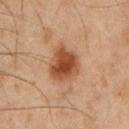{
  "biopsy_status": "not biopsied; imaged during a skin examination",
  "site": "front of the torso",
  "lighting": "cross-polarized",
  "lesion_size": {
    "long_diameter_mm_approx": 4.5
  },
  "patient": {
    "sex": "male",
    "age_approx": 60
  },
  "image": {
    "source": "total-body photography crop",
    "field_of_view_mm": 15
  }
}